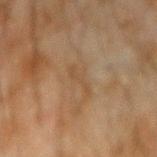Notes:
– workup — imaged on a skin check; not biopsied
– subject — male, in their mid-40s
– acquisition — ~15 mm tile from a whole-body skin photo
– lesion size — ≈4 mm
– anatomic site — the left forearm